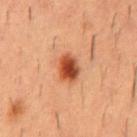Measured at roughly 3.5 mm in maximum diameter.
On the mid back.
The lesion-visualizer software estimated a border-irregularity rating of about 2/10, internal color variation of about 6.5 on a 0–10 scale, and radial color variation of about 2. The software also gave a classifier nevus-likeness of about 100/100 and a lesion-detection confidence of about 100/100.
A male subject roughly 55 years of age.
A 15 mm close-up tile from a total-body photography series done for melanoma screening.